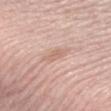Clinical impression:
Captured during whole-body skin photography for melanoma surveillance; the lesion was not biopsied.
Background:
Cropped from a total-body skin-imaging series; the visible field is about 15 mm. From the left forearm. A female subject, aged approximately 40.